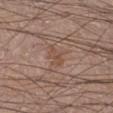Findings:
– body site — the right lower leg
– lesion diameter — ~2.5 mm (longest diameter)
– tile lighting — white-light
– patient — male, about 20 years old
– image source — 15 mm crop, total-body photography
– automated metrics — a footprint of about 3.5 mm², a shape eccentricity near 0.8, and a symmetry-axis asymmetry near 0.25; a border-irregularity rating of about 2.5/10, internal color variation of about 2 on a 0–10 scale, and a peripheral color-asymmetry measure near 0.5